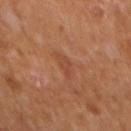- biopsy status · imaged on a skin check; not biopsied
- patient · male, aged around 65
- size · ≈3 mm
- image source · total-body-photography crop, ~15 mm field of view
- anatomic site · the mid back